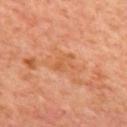No biopsy was performed on this lesion — it was imaged during a full skin examination and was not determined to be concerning.
The lesion is on the mid back.
Measured at roughly 3 mm in maximum diameter.
A male patient, in their mid-60s.
The tile uses cross-polarized illumination.
A region of skin cropped from a whole-body photographic capture, roughly 15 mm wide.